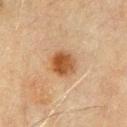Clinical impression: No biopsy was performed on this lesion — it was imaged during a full skin examination and was not determined to be concerning. Image and clinical context: On the chest. The subject is a male aged 68 to 72. Approximately 3 mm at its widest. A lesion tile, about 15 mm wide, cut from a 3D total-body photograph. The tile uses cross-polarized illumination.